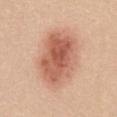Q: Was a biopsy performed?
A: no biopsy performed (imaged during a skin exam)
Q: Automated lesion metrics?
A: a border-irregularity rating of about 1.5/10, a color-variation rating of about 6/10, and peripheral color asymmetry of about 2; a classifier nevus-likeness of about 100/100 and lesion-presence confidence of about 100/100
Q: How was this image acquired?
A: ~15 mm tile from a whole-body skin photo
Q: Who is the patient?
A: female, aged approximately 20
Q: What is the anatomic site?
A: the chest
Q: How was the tile lit?
A: white-light illumination